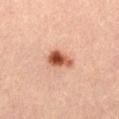<lesion>
  <image>
    <source>total-body photography crop</source>
    <field_of_view_mm>15</field_of_view_mm>
  </image>
  <site>left thigh</site>
  <patient>
    <sex>female</sex>
    <age_approx>45</age_approx>
  </patient>
</lesion>